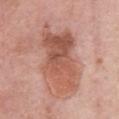Q: Was this lesion biopsied?
A: total-body-photography surveillance lesion; no biopsy
Q: Lesion location?
A: the chest
Q: What lighting was used for the tile?
A: white-light
Q: What did automated image analysis measure?
A: a lesion area of about 32 mm², an eccentricity of roughly 0.85, and a shape-asymmetry score of about 0.35 (0 = symmetric); a mean CIELAB color near L≈56 a*≈25 b*≈29; a border-irregularity rating of about 4.5/10, a within-lesion color-variation index near 7/10, and peripheral color asymmetry of about 2.5; a classifier nevus-likeness of about 80/100
Q: What is the lesion's diameter?
A: about 9.5 mm
Q: Patient demographics?
A: female, aged around 75
Q: What is the imaging modality?
A: 15 mm crop, total-body photography